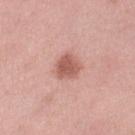{
  "biopsy_status": "not biopsied; imaged during a skin examination",
  "lesion_size": {
    "long_diameter_mm_approx": 2.5
  },
  "site": "leg",
  "patient": {
    "sex": "female",
    "age_approx": 40
  },
  "lighting": "white-light",
  "image": {
    "source": "total-body photography crop",
    "field_of_view_mm": 15
  }
}